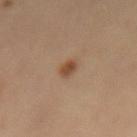{
  "biopsy_status": "not biopsied; imaged during a skin examination",
  "image": {
    "source": "total-body photography crop",
    "field_of_view_mm": 15
  },
  "patient": {
    "sex": "male",
    "age_approx": 65
  },
  "site": "lower back"
}